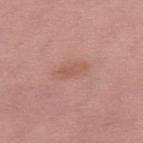This lesion was catalogued during total-body skin photography and was not selected for biopsy. Approximately 4.5 mm at its widest. The lesion is located on the right thigh. Cropped from a whole-body photographic skin survey; the tile spans about 15 mm. The patient is a female approximately 40 years of age.